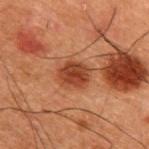Impression:
No biopsy was performed on this lesion — it was imaged during a full skin examination and was not determined to be concerning.
Acquisition and patient details:
From the upper back. A close-up tile cropped from a whole-body skin photograph, about 15 mm across. Automated tile analysis of the lesion measured a footprint of about 6 mm², a shape eccentricity near 0.45, and two-axis asymmetry of about 0.2. The software also gave a mean CIELAB color near L≈31 a*≈23 b*≈28 and a lesion–skin lightness drop of about 10. The analysis additionally found border irregularity of about 1.5 on a 0–10 scale, internal color variation of about 3 on a 0–10 scale, and a peripheral color-asymmetry measure near 1. The software also gave a lesion-detection confidence of about 100/100. The tile uses cross-polarized illumination. A male subject roughly 50 years of age. Measured at roughly 3 mm in maximum diameter.